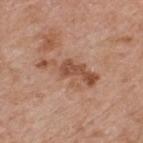follow-up=no biopsy performed (imaged during a skin exam)
patient=male, aged 58 to 62
image source=total-body-photography crop, ~15 mm field of view
location=the upper back
lesion diameter=~7 mm (longest diameter)
TBP lesion metrics=an average lesion color of about L≈52 a*≈22 b*≈31 (CIELAB) and a normalized lesion–skin contrast near 7.5; an automated nevus-likeness rating near 15 out of 100